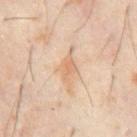The lesion was tiled from a total-body skin photograph and was not biopsied. Longest diameter approximately 5 mm. This is a cross-polarized tile. Cropped from a whole-body photographic skin survey; the tile spans about 15 mm. An algorithmic analysis of the crop reported border irregularity of about 5.5 on a 0–10 scale, a color-variation rating of about 2/10, and radial color variation of about 0.5. A male patient, aged approximately 60. From the abdomen.